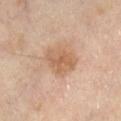The lesion was tiled from a total-body skin photograph and was not biopsied. The lesion is located on the leg. The patient is a male about 55 years old. Cropped from a whole-body photographic skin survey; the tile spans about 15 mm. Imaged with cross-polarized lighting.